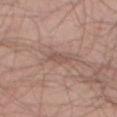Clinical impression: This lesion was catalogued during total-body skin photography and was not selected for biopsy. Background: A male subject in their 40s. The lesion is on the left thigh. Captured under white-light illumination. Longest diameter approximately 3 mm. A region of skin cropped from a whole-body photographic capture, roughly 15 mm wide.